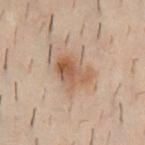Recorded during total-body skin imaging; not selected for excision or biopsy. A 15 mm close-up extracted from a 3D total-body photography capture. On the chest. The patient is a male aged 28–32.